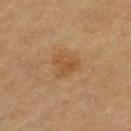| field | value |
|---|---|
| follow-up | catalogued during a skin exam; not biopsied |
| site | the left upper arm |
| illumination | cross-polarized illumination |
| patient | female, aged approximately 80 |
| acquisition | 15 mm crop, total-body photography |
| image-analysis metrics | a lesion color around L≈43 a*≈17 b*≈33 in CIELAB and a lesion-to-skin contrast of about 6 (normalized; higher = more distinct); a nevus-likeness score of about 5/100 |